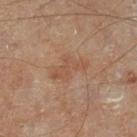Q: Was a biopsy performed?
A: total-body-photography surveillance lesion; no biopsy
Q: Patient demographics?
A: in their mid- to late 50s
Q: What kind of image is this?
A: ~15 mm crop, total-body skin-cancer survey
Q: How was the tile lit?
A: cross-polarized
Q: What is the anatomic site?
A: the left lower leg